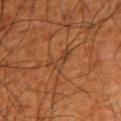No biopsy was performed on this lesion — it was imaged during a full skin examination and was not determined to be concerning. An algorithmic analysis of the crop reported a lesion area of about 4.5 mm² and an outline eccentricity of about 0.9 (0 = round, 1 = elongated). The software also gave a border-irregularity index near 6/10, a within-lesion color-variation index near 3/10, and peripheral color asymmetry of about 0.5. A male subject, in their mid- to late 60s. A close-up tile cropped from a whole-body skin photograph, about 15 mm across. Located on the left upper arm. This is a cross-polarized tile.